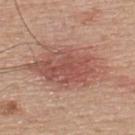<lesion>
  <lighting>white-light</lighting>
  <lesion_size>
    <long_diameter_mm_approx>10.0</long_diameter_mm_approx>
  </lesion_size>
  <image>
    <source>total-body photography crop</source>
    <field_of_view_mm>15</field_of_view_mm>
  </image>
  <automated_metrics>
    <area_mm2_approx>35.0</area_mm2_approx>
    <eccentricity>0.8</eccentricity>
    <shape_asymmetry>0.25</shape_asymmetry>
    <nevus_likeness_0_100>75</nevus_likeness_0_100>
  </automated_metrics>
  <patient>
    <sex>male</sex>
    <age_approx>55</age_approx>
  </patient>
  <site>upper back</site>
</lesion>